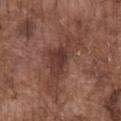Captured during whole-body skin photography for melanoma surveillance; the lesion was not biopsied. The subject is a male aged 73–77. The total-body-photography lesion software estimated a footprint of about 15 mm², an eccentricity of roughly 0.9, and two-axis asymmetry of about 0.55. And it measured an average lesion color of about L≈38 a*≈20 b*≈24 (CIELAB), about 9 CIELAB-L* units darker than the surrounding skin, and a normalized lesion–skin contrast near 7.5. It also reported a border-irregularity index near 8/10, internal color variation of about 3.5 on a 0–10 scale, and radial color variation of about 1. The recorded lesion diameter is about 7.5 mm. Located on the chest. A lesion tile, about 15 mm wide, cut from a 3D total-body photograph.